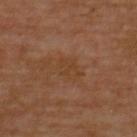Part of a total-body skin-imaging series; this lesion was reviewed on a skin check and was not flagged for biopsy.
The lesion is on the upper back.
The patient is a male roughly 55 years of age.
Measured at roughly 3 mm in maximum diameter.
A close-up tile cropped from a whole-body skin photograph, about 15 mm across.